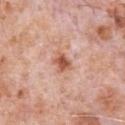Clinical impression: Imaged during a routine full-body skin examination; the lesion was not biopsied and no histopathology is available. Context: The patient is a male aged approximately 80. About 3 mm across. This is a white-light tile. Cropped from a whole-body photographic skin survey; the tile spans about 15 mm. The lesion is located on the chest.